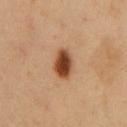patient: male, roughly 50 years of age
image: ~15 mm crop, total-body skin-cancer survey
anatomic site: the chest
lesion size: ~3.5 mm (longest diameter)